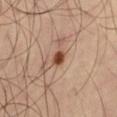No biopsy was performed on this lesion — it was imaged during a full skin examination and was not determined to be concerning.
Cropped from a whole-body photographic skin survey; the tile spans about 15 mm.
The lesion is on the left thigh.
This is a cross-polarized tile.
Automated tile analysis of the lesion measured an area of roughly 2.5 mm², a shape eccentricity near 0.35, and a symmetry-axis asymmetry near 0.2. The analysis additionally found a border-irregularity rating of about 1.5/10 and internal color variation of about 1.5 on a 0–10 scale. The analysis additionally found a detector confidence of about 100 out of 100 that the crop contains a lesion.
A male patient in their mid-60s.
Longest diameter approximately 2 mm.